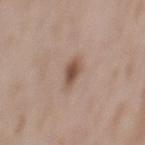Clinical impression: Part of a total-body skin-imaging series; this lesion was reviewed on a skin check and was not flagged for biopsy. Clinical summary: The patient is a male roughly 55 years of age. Captured under white-light illumination. On the back. A roughly 15 mm field-of-view crop from a total-body skin photograph.